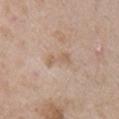<record>
  <biopsy_status>not biopsied; imaged during a skin examination</biopsy_status>
</record>